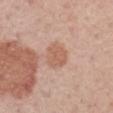<tbp_lesion>
<patient>
  <sex>male</sex>
  <age_approx>60</age_approx>
</patient>
<site>right upper arm</site>
<lesion_size>
  <long_diameter_mm_approx>3.0</long_diameter_mm_approx>
</lesion_size>
<image>
  <source>total-body photography crop</source>
  <field_of_view_mm>15</field_of_view_mm>
</image>
</tbp_lesion>